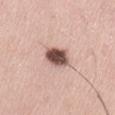Part of a total-body skin-imaging series; this lesion was reviewed on a skin check and was not flagged for biopsy.
The lesion is on the left thigh.
The lesion-visualizer software estimated an area of roughly 6.5 mm², an eccentricity of roughly 0.55, and a symmetry-axis asymmetry near 0.15. It also reported a lesion color around L≈51 a*≈20 b*≈23 in CIELAB, a lesion–skin lightness drop of about 22, and a normalized lesion–skin contrast near 13.5. The analysis additionally found a border-irregularity index near 1.5/10. The software also gave an automated nevus-likeness rating near 70 out of 100 and a detector confidence of about 100 out of 100 that the crop contains a lesion.
The lesion's longest dimension is about 3 mm.
A male patient approximately 55 years of age.
Captured under white-light illumination.
A 15 mm close-up tile from a total-body photography series done for melanoma screening.